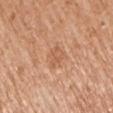Q: Was a biopsy performed?
A: imaged on a skin check; not biopsied
Q: What are the patient's age and sex?
A: female, aged 73 to 77
Q: What did automated image analysis measure?
A: an area of roughly 4 mm², an eccentricity of roughly 0.75, and a symmetry-axis asymmetry near 0.3; a border-irregularity index near 3.5/10, internal color variation of about 2 on a 0–10 scale, and peripheral color asymmetry of about 0.5
Q: Where on the body is the lesion?
A: the right upper arm
Q: How large is the lesion?
A: ≈3 mm
Q: What is the imaging modality?
A: total-body-photography crop, ~15 mm field of view